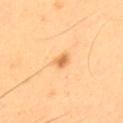Clinical summary: The tile uses cross-polarized illumination. Automated image analysis of the tile measured a shape eccentricity near 0.8. And it measured an average lesion color of about L≈71 a*≈22 b*≈44 (CIELAB), roughly 9 lightness units darker than nearby skin, and a lesion-to-skin contrast of about 5 (normalized; higher = more distinct). A male patient, aged around 55. The lesion is located on the back. A 15 mm close-up tile from a total-body photography series done for melanoma screening.